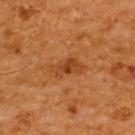patient:
  sex: male
  age_approx: 65
lighting: cross-polarized
site: upper back
image:
  source: total-body photography crop
  field_of_view_mm: 15